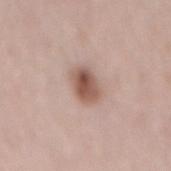– workup — catalogued during a skin exam; not biopsied
– body site — the mid back
– automated metrics — a border-irregularity rating of about 1.5/10, a within-lesion color-variation index near 6/10, and radial color variation of about 2; lesion-presence confidence of about 100/100
– patient — female, aged around 30
– illumination — white-light illumination
– acquisition — total-body-photography crop, ~15 mm field of view